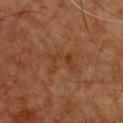Assessment: The lesion was tiled from a total-body skin photograph and was not biopsied. Acquisition and patient details: This is a cross-polarized tile. The total-body-photography lesion software estimated an outline eccentricity of about 0.75 (0 = round, 1 = elongated). The analysis additionally found a mean CIELAB color near L≈31 a*≈19 b*≈28 and a lesion-to-skin contrast of about 5 (normalized; higher = more distinct). A male patient approximately 60 years of age. Located on the chest. Cropped from a whole-body photographic skin survey; the tile spans about 15 mm. Measured at roughly 4 mm in maximum diameter.